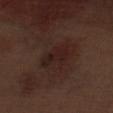The lesion was photographed on a routine skin check and not biopsied; there is no pathology result. This is a white-light tile. The lesion is located on the abdomen. A male patient in their 70s. A 15 mm close-up tile from a total-body photography series done for melanoma screening. An algorithmic analysis of the crop reported an area of roughly 8 mm² and a shape-asymmetry score of about 0.4 (0 = symmetric). Measured at roughly 4.5 mm in maximum diameter.